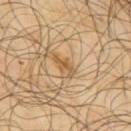Captured during whole-body skin photography for melanoma surveillance; the lesion was not biopsied. A male patient, about 65 years old. A close-up tile cropped from a whole-body skin photograph, about 15 mm across. Located on the upper back. Automated tile analysis of the lesion measured about 9 CIELAB-L* units darker than the surrounding skin. It also reported internal color variation of about 0 on a 0–10 scale. And it measured an automated nevus-likeness rating near 30 out of 100 and lesion-presence confidence of about 70/100. The tile uses cross-polarized illumination.